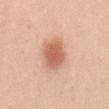Assessment: No biopsy was performed on this lesion — it was imaged during a full skin examination and was not determined to be concerning. Clinical summary: The patient is a female about 20 years old. The tile uses white-light illumination. The recorded lesion diameter is about 4 mm. This image is a 15 mm lesion crop taken from a total-body photograph. On the lower back.